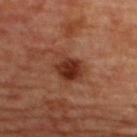Clinical impression:
Captured during whole-body skin photography for melanoma surveillance; the lesion was not biopsied.
Context:
Approximately 4 mm at its widest. A lesion tile, about 15 mm wide, cut from a 3D total-body photograph. The lesion-visualizer software estimated a lesion area of about 10 mm², an outline eccentricity of about 0.6 (0 = round, 1 = elongated), and two-axis asymmetry of about 0.25. The analysis additionally found an average lesion color of about L≈34 a*≈24 b*≈29 (CIELAB) and a lesion–skin lightness drop of about 11. It also reported a border-irregularity index near 2.5/10 and a color-variation rating of about 6/10. And it measured a nevus-likeness score of about 95/100 and a lesion-detection confidence of about 100/100. The lesion is on the upper back. A female patient approximately 45 years of age.